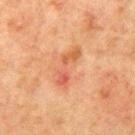Q: Is there a histopathology result?
A: no biopsy performed (imaged during a skin exam)
Q: Who is the patient?
A: male, aged 68–72
Q: What is the imaging modality?
A: total-body-photography crop, ~15 mm field of view
Q: Lesion location?
A: the mid back